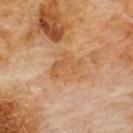The lesion was photographed on a routine skin check and not biopsied; there is no pathology result.
A roughly 15 mm field-of-view crop from a total-body skin photograph.
This is a cross-polarized tile.
From the chest.
The lesion's longest dimension is about 4 mm.
An algorithmic analysis of the crop reported a shape eccentricity near 0.7 and two-axis asymmetry of about 0.4. The software also gave roughly 6 lightness units darker than nearby skin and a lesion-to-skin contrast of about 6 (normalized; higher = more distinct).
The subject is a male aged 58 to 62.